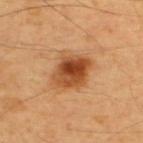notes = catalogued during a skin exam; not biopsied | image source = total-body-photography crop, ~15 mm field of view | subject = male, roughly 55 years of age | location = the upper back | tile lighting = cross-polarized illumination.